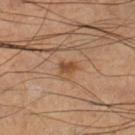Clinical impression: Part of a total-body skin-imaging series; this lesion was reviewed on a skin check and was not flagged for biopsy. Acquisition and patient details: This image is a 15 mm lesion crop taken from a total-body photograph. Located on the left lower leg. The recorded lesion diameter is about 2 mm. A male patient, aged approximately 60.